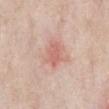<case>
<biopsy_status>not biopsied; imaged during a skin examination</biopsy_status>
<automated_metrics>
  <area_mm2_approx>5.0</area_mm2_approx>
  <eccentricity>0.65</eccentricity>
  <shape_asymmetry>0.2</shape_asymmetry>
  <border_irregularity_0_10>2.0</border_irregularity_0_10>
  <color_variation_0_10>2.0</color_variation_0_10>
</automated_metrics>
<lighting>white-light</lighting>
<site>front of the torso</site>
<lesion_size>
  <long_diameter_mm_approx>3.0</long_diameter_mm_approx>
</lesion_size>
<patient>
  <sex>male</sex>
  <age_approx>60</age_approx>
</patient>
<image>
  <source>total-body photography crop</source>
  <field_of_view_mm>15</field_of_view_mm>
</image>
</case>